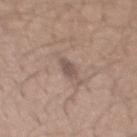- notes: total-body-photography surveillance lesion; no biopsy
- patient: male, roughly 25 years of age
- image-analysis metrics: a border-irregularity rating of about 2/10 and radial color variation of about 0.5
- image: total-body-photography crop, ~15 mm field of view
- diameter: ≈2.5 mm
- body site: the mid back
- lighting: white-light